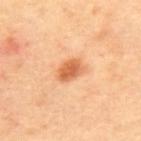<tbp_lesion>
<biopsy_status>not biopsied; imaged during a skin examination</biopsy_status>
<automated_metrics>
  <eccentricity>0.75</eccentricity>
</automated_metrics>
<patient>
  <sex>female</sex>
  <age_approx>40</age_approx>
</patient>
<lighting>cross-polarized</lighting>
<image>
  <source>total-body photography crop</source>
  <field_of_view_mm>15</field_of_view_mm>
</image>
<lesion_size>
  <long_diameter_mm_approx>3.0</long_diameter_mm_approx>
</lesion_size>
<site>back</site>
</tbp_lesion>